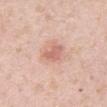Imaged during a routine full-body skin examination; the lesion was not biopsied and no histopathology is available.
On the right upper arm.
Imaged with white-light lighting.
A lesion tile, about 15 mm wide, cut from a 3D total-body photograph.
A male subject about 40 years old.
Measured at roughly 3.5 mm in maximum diameter.